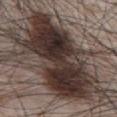This lesion was catalogued during total-body skin photography and was not selected for biopsy. Located on the abdomen. This is a white-light tile. An algorithmic analysis of the crop reported a footprint of about 60 mm² and an eccentricity of roughly 0.9. And it measured about 18 CIELAB-L* units darker than the surrounding skin and a normalized border contrast of about 15. The analysis additionally found a border-irregularity index near 6.5/10, a color-variation rating of about 8.5/10, and a peripheral color-asymmetry measure near 3. A male subject approximately 65 years of age. A region of skin cropped from a whole-body photographic capture, roughly 15 mm wide.